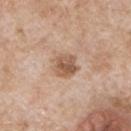Background: On the front of the torso. A male patient, approximately 65 years of age. A close-up tile cropped from a whole-body skin photograph, about 15 mm across.